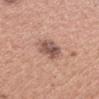The lesion is located on the head or neck.
A male subject, aged approximately 30.
A region of skin cropped from a whole-body photographic capture, roughly 15 mm wide.